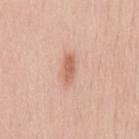<record>
<biopsy_status>not biopsied; imaged during a skin examination</biopsy_status>
<lighting>white-light</lighting>
<site>chest</site>
<lesion_size>
  <long_diameter_mm_approx>3.5</long_diameter_mm_approx>
</lesion_size>
<image>
  <source>total-body photography crop</source>
  <field_of_view_mm>15</field_of_view_mm>
</image>
<automated_metrics>
  <cielab_L>63</cielab_L>
  <cielab_a>24</cielab_a>
  <cielab_b>31</cielab_b>
  <vs_skin_darker_L>11.0</vs_skin_darker_L>
  <vs_skin_contrast_norm>7.0</vs_skin_contrast_norm>
  <nevus_likeness_0_100>95</nevus_likeness_0_100>
  <lesion_detection_confidence_0_100>100</lesion_detection_confidence_0_100>
</automated_metrics>
<patient>
  <sex>male</sex>
  <age_approx>60</age_approx>
</patient>
</record>